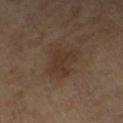This lesion was catalogued during total-body skin photography and was not selected for biopsy. A lesion tile, about 15 mm wide, cut from a 3D total-body photograph. A male subject, about 70 years old. The recorded lesion diameter is about 4.5 mm. The lesion is located on the arm. Automated image analysis of the tile measured a mean CIELAB color near L≈32 a*≈14 b*≈24, about 6 CIELAB-L* units darker than the surrounding skin, and a lesion-to-skin contrast of about 6 (normalized; higher = more distinct). The analysis additionally found a classifier nevus-likeness of about 5/100 and a detector confidence of about 100 out of 100 that the crop contains a lesion.